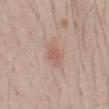No biopsy was performed on this lesion — it was imaged during a full skin examination and was not determined to be concerning. A 15 mm close-up extracted from a 3D total-body photography capture. The lesion is on the abdomen. Automated tile analysis of the lesion measured a footprint of about 4 mm², a shape eccentricity near 0.85, and a symmetry-axis asymmetry near 0.25. The software also gave a mean CIELAB color near L≈58 a*≈20 b*≈26, a lesion–skin lightness drop of about 8, and a normalized border contrast of about 5.5. The analysis additionally found an automated nevus-likeness rating near 20 out of 100 and a lesion-detection confidence of about 100/100. About 3 mm across. A male subject aged around 60.